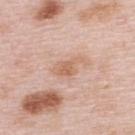Assessment:
The lesion was tiled from a total-body skin photograph and was not biopsied.
Image and clinical context:
On the back. About 4 mm across. A roughly 15 mm field-of-view crop from a total-body skin photograph. The patient is a female aged 63 to 67. Imaged with white-light lighting.